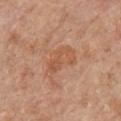This lesion was catalogued during total-body skin photography and was not selected for biopsy. The total-body-photography lesion software estimated a footprint of about 9 mm² and a symmetry-axis asymmetry near 0.35. And it measured a border-irregularity index near 4/10, a within-lesion color-variation index near 3/10, and a peripheral color-asymmetry measure near 1.5. The software also gave a nevus-likeness score of about 0/100 and a lesion-detection confidence of about 100/100. On the chest. A lesion tile, about 15 mm wide, cut from a 3D total-body photograph. The patient is a male aged around 60.